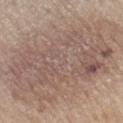The tile uses white-light illumination. The lesion is located on the left lower leg. About 17 mm across. A male subject aged approximately 70. Automated tile analysis of the lesion measured an area of roughly 125 mm², a shape eccentricity near 0.85, and two-axis asymmetry of about 0.15. The software also gave an average lesion color of about L≈56 a*≈15 b*≈24 (CIELAB) and a normalized border contrast of about 6.5. It also reported a color-variation rating of about 5.5/10 and peripheral color asymmetry of about 2. A roughly 15 mm field-of-view crop from a total-body skin photograph.